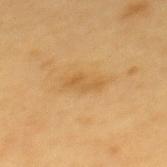Acquisition and patient details: A male patient, aged 58 to 62. Located on the back. The total-body-photography lesion software estimated a lesion color around L≈58 a*≈19 b*≈44 in CIELAB and a lesion–skin lightness drop of about 7. The analysis additionally found a border-irregularity index near 5/10. And it measured a classifier nevus-likeness of about 0/100 and lesion-presence confidence of about 100/100. The recorded lesion diameter is about 3.5 mm. This is a cross-polarized tile. A roughly 15 mm field-of-view crop from a total-body skin photograph.